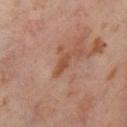Clinical impression:
Recorded during total-body skin imaging; not selected for excision or biopsy.
Background:
This is a cross-polarized tile. Longest diameter approximately 3 mm. A 15 mm close-up extracted from a 3D total-body photography capture. The patient is a female in their mid- to late 50s. Located on the right thigh.